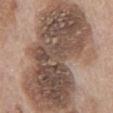Impression: Recorded during total-body skin imaging; not selected for excision or biopsy. Background: Cropped from a whole-body photographic skin survey; the tile spans about 15 mm. From the chest. Longest diameter approximately 16 mm. Imaged with white-light lighting. An algorithmic analysis of the crop reported a footprint of about 85 mm² and an eccentricity of roughly 0.9. A male patient, in their 70s.